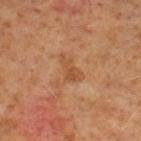Assessment:
Recorded during total-body skin imaging; not selected for excision or biopsy.
Clinical summary:
A 15 mm close-up tile from a total-body photography series done for melanoma screening. This is a cross-polarized tile. Approximately 3.5 mm at its widest. Located on the right lower leg. A male patient, roughly 60 years of age. An algorithmic analysis of the crop reported an average lesion color of about L≈47 a*≈22 b*≈35 (CIELAB), roughly 7 lightness units darker than nearby skin, and a normalized border contrast of about 6.